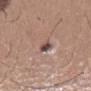Captured during whole-body skin photography for melanoma surveillance; the lesion was not biopsied.
A 15 mm close-up extracted from a 3D total-body photography capture.
A male patient aged 63 to 67.
The lesion is on the mid back.
The recorded lesion diameter is about 3.5 mm.